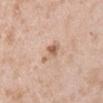follow-up: total-body-photography surveillance lesion; no biopsy
lesion diameter: ≈3 mm
image-analysis metrics: an area of roughly 4.5 mm², a shape eccentricity near 0.8, and two-axis asymmetry of about 0.4; a border-irregularity rating of about 4.5/10, internal color variation of about 5 on a 0–10 scale, and peripheral color asymmetry of about 1.5; a classifier nevus-likeness of about 50/100 and a lesion-detection confidence of about 100/100
location: the left upper arm
image source: total-body-photography crop, ~15 mm field of view
illumination: white-light illumination
subject: male, aged around 50